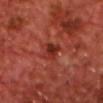Approximately 3 mm at its widest. Captured under cross-polarized illumination. The lesion is located on the chest. A region of skin cropped from a whole-body photographic capture, roughly 15 mm wide. A male patient aged 68–72.